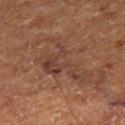Cropped from a whole-body photographic skin survey; the tile spans about 15 mm.
From the right thigh.
A male patient aged approximately 75.